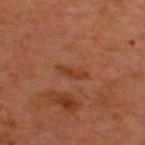Case summary:
– workup: imaged on a skin check; not biopsied
– lesion size: about 3.5 mm
– site: the upper back
– patient: male, aged around 50
– image source: ~15 mm tile from a whole-body skin photo
– image-analysis metrics: a mean CIELAB color near L≈40 a*≈26 b*≈35 and a lesion-to-skin contrast of about 6.5 (normalized; higher = more distinct); a border-irregularity rating of about 4.5/10 and radial color variation of about 0